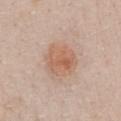tile lighting: white-light illumination | patient: female, in their mid- to late 30s | size: ≈4.5 mm | automated lesion analysis: an outline eccentricity of about 0.45 (0 = round, 1 = elongated) and a symmetry-axis asymmetry near 0.15; peripheral color asymmetry of about 1.5 | site: the chest | image: ~15 mm crop, total-body skin-cancer survey.